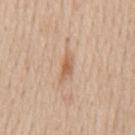Recorded during total-body skin imaging; not selected for excision or biopsy. A male subject, in their 60s. The lesion is located on the back. A lesion tile, about 15 mm wide, cut from a 3D total-body photograph. Automated tile analysis of the lesion measured a footprint of about 4.5 mm² and a shape eccentricity near 0.9. The analysis additionally found a border-irregularity rating of about 3.5/10, a within-lesion color-variation index near 3.5/10, and peripheral color asymmetry of about 1. The software also gave a classifier nevus-likeness of about 15/100 and a detector confidence of about 100 out of 100 that the crop contains a lesion. The lesion's longest dimension is about 3.5 mm.